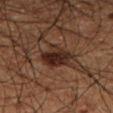Imaged during a routine full-body skin examination; the lesion was not biopsied and no histopathology is available. Longest diameter approximately 3.5 mm. The total-body-photography lesion software estimated an area of roughly 8 mm², an outline eccentricity of about 0.55 (0 = round, 1 = elongated), and a symmetry-axis asymmetry near 0.2. It also reported border irregularity of about 2 on a 0–10 scale, a within-lesion color-variation index near 4/10, and a peripheral color-asymmetry measure near 1. This is a cross-polarized tile. A male patient, aged 58–62. Located on the leg. A roughly 15 mm field-of-view crop from a total-body skin photograph.